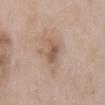biopsy status=no biopsy performed (imaged during a skin exam) | acquisition=total-body-photography crop, ~15 mm field of view | TBP lesion metrics=a lesion color around L≈56 a*≈16 b*≈28 in CIELAB, a lesion–skin lightness drop of about 9, and a normalized border contrast of about 6.5; a border-irregularity index near 3.5/10, a color-variation rating of about 4/10, and a peripheral color-asymmetry measure near 1.5; an automated nevus-likeness rating near 0 out of 100 and a lesion-detection confidence of about 100/100 | diameter=about 3.5 mm | lighting=white-light illumination | patient=male, roughly 65 years of age | site=the mid back.